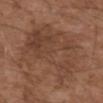Recorded during total-body skin imaging; not selected for excision or biopsy. A male subject aged approximately 75. Approximately 11 mm at its widest. Imaged with white-light lighting. A lesion tile, about 15 mm wide, cut from a 3D total-body photograph. The lesion is located on the upper back.